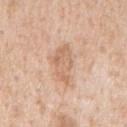{
  "patient": {
    "sex": "male",
    "age_approx": 65
  },
  "lighting": "white-light",
  "site": "mid back",
  "image": {
    "source": "total-body photography crop",
    "field_of_view_mm": 15
  },
  "automated_metrics": {
    "cielab_L": 66,
    "cielab_a": 19,
    "cielab_b": 32,
    "vs_skin_darker_L": 9.0,
    "vs_skin_contrast_norm": 5.5,
    "border_irregularity_0_10": 4.0,
    "color_variation_0_10": 3.5,
    "peripheral_color_asymmetry": 1.0
  }
}